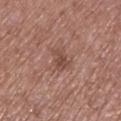* follow-up · total-body-photography surveillance lesion; no biopsy
* patient · female, approximately 75 years of age
* anatomic site · the left lower leg
* tile lighting · white-light illumination
* image · 15 mm crop, total-body photography
* lesion size · ~3 mm (longest diameter)